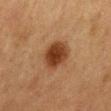| key | value |
|---|---|
| biopsy status | catalogued during a skin exam; not biopsied |
| size | ~4 mm (longest diameter) |
| image source | ~15 mm crop, total-body skin-cancer survey |
| lighting | cross-polarized |
| subject | male, aged 73 to 77 |
| body site | the mid back |